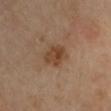Clinical impression:
This lesion was catalogued during total-body skin photography and was not selected for biopsy.
Background:
A region of skin cropped from a whole-body photographic capture, roughly 15 mm wide. From the left upper arm. Measured at roughly 3 mm in maximum diameter. The tile uses cross-polarized illumination. Automated tile analysis of the lesion measured a mean CIELAB color near L≈44 a*≈19 b*≈32 and a normalized border contrast of about 7.5. The software also gave an automated nevus-likeness rating near 65 out of 100 and a detector confidence of about 100 out of 100 that the crop contains a lesion.